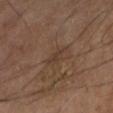Q: Was a biopsy performed?
A: no biopsy performed (imaged during a skin exam)
Q: What lighting was used for the tile?
A: cross-polarized
Q: Lesion size?
A: about 3 mm
Q: What kind of image is this?
A: total-body-photography crop, ~15 mm field of view
Q: Patient demographics?
A: male, aged around 70
Q: Lesion location?
A: the left lower leg